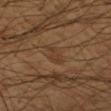The lesion is located on the left forearm.
The total-body-photography lesion software estimated an automated nevus-likeness rating near 0 out of 100 and a lesion-detection confidence of about 55/100.
A lesion tile, about 15 mm wide, cut from a 3D total-body photograph.
Longest diameter approximately 2.5 mm.
A male subject, in their mid-60s.